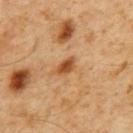follow-up — imaged on a skin check; not biopsied | automated metrics — a footprint of about 4 mm², an eccentricity of roughly 0.8, and a symmetry-axis asymmetry near 0.25; a mean CIELAB color near L≈46 a*≈23 b*≈37 and a lesion-to-skin contrast of about 9 (normalized; higher = more distinct); a border-irregularity rating of about 2/10, internal color variation of about 3 on a 0–10 scale, and radial color variation of about 1; an automated nevus-likeness rating near 90 out of 100 and a lesion-detection confidence of about 100/100 | acquisition — ~15 mm crop, total-body skin-cancer survey | location — the back | patient — male, in their 60s | tile lighting — cross-polarized.